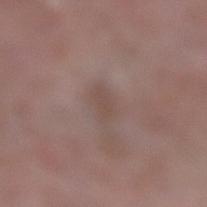{"biopsy_status": "not biopsied; imaged during a skin examination", "site": "left lower leg", "lesion_size": {"long_diameter_mm_approx": 2.5}, "image": {"source": "total-body photography crop", "field_of_view_mm": 15}, "automated_metrics": {"eccentricity": 0.8, "shape_asymmetry": 0.25, "cielab_L": 47, "cielab_a": 16, "cielab_b": 21, "vs_skin_darker_L": 6.0, "border_irregularity_0_10": 2.5, "color_variation_0_10": 1.5, "peripheral_color_asymmetry": 0.5}, "patient": {"sex": "female", "age_approx": 70}, "lighting": "white-light"}